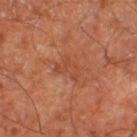Background:
This is a cross-polarized tile. This image is a 15 mm lesion crop taken from a total-body photograph. The lesion is located on the right thigh. Longest diameter approximately 3.5 mm. The patient is about 65 years old.